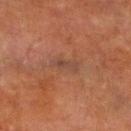Impression: This lesion was catalogued during total-body skin photography and was not selected for biopsy. Background: A 15 mm crop from a total-body photograph taken for skin-cancer surveillance. Captured under cross-polarized illumination. The patient is a male aged 68 to 72. The lesion is on the right lower leg.